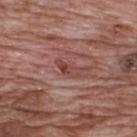acquisition = ~15 mm crop, total-body skin-cancer survey
site = the upper back
subject = male, aged around 70